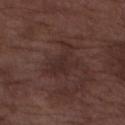| key | value |
|---|---|
| notes | total-body-photography surveillance lesion; no biopsy |